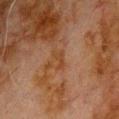Imaged during a routine full-body skin examination; the lesion was not biopsied and no histopathology is available.
Longest diameter approximately 2.5 mm.
Imaged with cross-polarized lighting.
A male subject aged 78 to 82.
The lesion-visualizer software estimated a lesion color around L≈34 a*≈19 b*≈29 in CIELAB, a lesion–skin lightness drop of about 5, and a normalized lesion–skin contrast near 6. And it measured a border-irregularity rating of about 5/10.
A 15 mm close-up tile from a total-body photography series done for melanoma screening.
Located on the upper back.